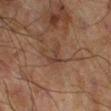{
  "biopsy_status": "not biopsied; imaged during a skin examination",
  "lesion_size": {
    "long_diameter_mm_approx": 3.0
  },
  "patient": {
    "sex": "male",
    "age_approx": 70
  },
  "site": "right lower leg",
  "lighting": "cross-polarized",
  "image": {
    "source": "total-body photography crop",
    "field_of_view_mm": 15
  }
}